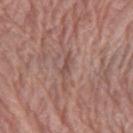follow-up=catalogued during a skin exam; not biopsied
subject=male, aged around 80
diameter=≈2.5 mm
TBP lesion metrics=a footprint of about 2.5 mm² and two-axis asymmetry of about 0.4; a border-irregularity index near 4/10, internal color variation of about 0 on a 0–10 scale, and radial color variation of about 0; an automated nevus-likeness rating near 0 out of 100
anatomic site=the left forearm
image source=~15 mm tile from a whole-body skin photo
lighting=white-light illumination